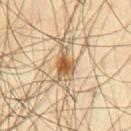Q: What did automated image analysis measure?
A: an average lesion color of about L≈59 a*≈16 b*≈37 (CIELAB) and roughly 14 lightness units darker than nearby skin
Q: What is the anatomic site?
A: the abdomen
Q: What is the lesion's diameter?
A: about 4 mm
Q: Illumination type?
A: cross-polarized
Q: What are the patient's age and sex?
A: male, in their mid- to late 60s
Q: How was this image acquired?
A: total-body-photography crop, ~15 mm field of view